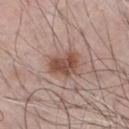{"biopsy_status": "not biopsied; imaged during a skin examination", "automated_metrics": {"area_mm2_approx": 8.0, "eccentricity": 0.7, "shape_asymmetry": 0.25, "cielab_L": 49, "cielab_a": 20, "cielab_b": 26, "vs_skin_darker_L": 12.0, "vs_skin_contrast_norm": 9.0, "border_irregularity_0_10": 3.0, "color_variation_0_10": 4.0, "peripheral_color_asymmetry": 1.5}, "site": "chest", "lesion_size": {"long_diameter_mm_approx": 4.0}, "lighting": "white-light", "image": {"source": "total-body photography crop", "field_of_view_mm": 15}, "patient": {"sex": "male", "age_approx": 65}}